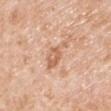The lesion was tiled from a total-body skin photograph and was not biopsied. From the right upper arm. Cropped from a whole-body photographic skin survey; the tile spans about 15 mm. The tile uses white-light illumination. The subject is a male in their mid-70s.